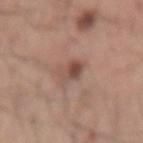Case summary:
- biopsy status — catalogued during a skin exam; not biopsied
- body site — the arm
- illumination — white-light illumination
- acquisition — ~15 mm crop, total-body skin-cancer survey
- size — ≈3 mm
- automated lesion analysis — an average lesion color of about L≈49 a*≈20 b*≈25 (CIELAB) and a normalized lesion–skin contrast near 7.5; a border-irregularity index near 2/10, internal color variation of about 6.5 on a 0–10 scale, and peripheral color asymmetry of about 2.5
- subject — male, roughly 60 years of age